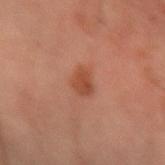Imaged during a routine full-body skin examination; the lesion was not biopsied and no histopathology is available. A roughly 15 mm field-of-view crop from a total-body skin photograph. A male subject, aged 58–62. The lesion is on the right forearm.